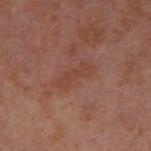The lesion was photographed on a routine skin check and not biopsied; there is no pathology result.
A male patient in their 30s.
About 4.5 mm across.
From the arm.
A 15 mm close-up tile from a total-body photography series done for melanoma screening.
Automated image analysis of the tile measured a lesion area of about 6 mm², an eccentricity of roughly 0.95, and a symmetry-axis asymmetry near 0.4. And it measured a lesion color around L≈34 a*≈19 b*≈23 in CIELAB and a lesion–skin lightness drop of about 4.